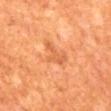Clinical impression: This lesion was catalogued during total-body skin photography and was not selected for biopsy. Clinical summary: A male subject aged around 65. The total-body-photography lesion software estimated a mean CIELAB color near L≈59 a*≈30 b*≈43, roughly 8 lightness units darker than nearby skin, and a normalized lesion–skin contrast near 5. Measured at roughly 3 mm in maximum diameter. From the mid back. A region of skin cropped from a whole-body photographic capture, roughly 15 mm wide. This is a cross-polarized tile.